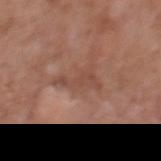Part of a total-body skin-imaging series; this lesion was reviewed on a skin check and was not flagged for biopsy.
The tile uses white-light illumination.
A 15 mm close-up extracted from a 3D total-body photography capture.
The lesion is on the mid back.
Longest diameter approximately 5 mm.
A male subject, aged around 70.